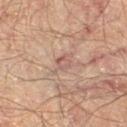The lesion is located on the left thigh. An algorithmic analysis of the crop reported a border-irregularity index near 5/10, a within-lesion color-variation index near 2.5/10, and radial color variation of about 0.5. A male subject, roughly 60 years of age. Longest diameter approximately 3.5 mm. Captured under cross-polarized illumination. A 15 mm close-up extracted from a 3D total-body photography capture.